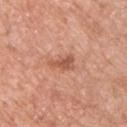Q: Was this lesion biopsied?
A: catalogued during a skin exam; not biopsied
Q: What did automated image analysis measure?
A: a border-irregularity rating of about 4/10, internal color variation of about 2 on a 0–10 scale, and peripheral color asymmetry of about 1; a nevus-likeness score of about 20/100 and lesion-presence confidence of about 100/100
Q: Illumination type?
A: white-light
Q: What are the patient's age and sex?
A: male, aged 53 to 57
Q: What kind of image is this?
A: ~15 mm crop, total-body skin-cancer survey
Q: What is the anatomic site?
A: the chest